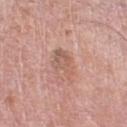Assessment: The lesion was tiled from a total-body skin photograph and was not biopsied. Background: The recorded lesion diameter is about 4.5 mm. This is a white-light tile. A lesion tile, about 15 mm wide, cut from a 3D total-body photograph. On the right thigh. A male subject, roughly 80 years of age.